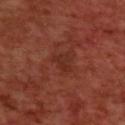Clinical summary: The lesion is located on the upper back. Cropped from a whole-body photographic skin survey; the tile spans about 15 mm. A male subject approximately 70 years of age. Captured under cross-polarized illumination. Automated tile analysis of the lesion measured a footprint of about 3.5 mm², a shape eccentricity near 0.7, and a shape-asymmetry score of about 0.4 (0 = symmetric). And it measured a border-irregularity rating of about 4/10 and internal color variation of about 0.5 on a 0–10 scale.